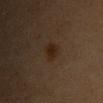Acquisition and patient details:
An algorithmic analysis of the crop reported an area of roughly 4.5 mm², an eccentricity of roughly 0.6, and a symmetry-axis asymmetry near 0.25. It also reported internal color variation of about 2 on a 0–10 scale and a peripheral color-asymmetry measure near 0.5. The software also gave a lesion-detection confidence of about 100/100. A 15 mm close-up extracted from a 3D total-body photography capture. Captured under cross-polarized illumination. A female patient about 45 years old. The lesion is located on the chest. The recorded lesion diameter is about 2.5 mm.